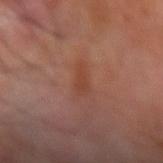Q: Is there a histopathology result?
A: imaged on a skin check; not biopsied
Q: Where on the body is the lesion?
A: the right forearm
Q: Patient demographics?
A: male, aged 68 to 72
Q: What kind of image is this?
A: ~15 mm crop, total-body skin-cancer survey
Q: What did automated image analysis measure?
A: a lesion area of about 3.5 mm² and an outline eccentricity of about 0.9 (0 = round, 1 = elongated); an average lesion color of about L≈41 a*≈24 b*≈29 (CIELAB), roughly 6 lightness units darker than nearby skin, and a normalized border contrast of about 6; a nevus-likeness score of about 0/100 and a lesion-detection confidence of about 100/100
Q: Illumination type?
A: cross-polarized illumination